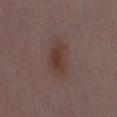{"biopsy_status": "not biopsied; imaged during a skin examination", "image": {"source": "total-body photography crop", "field_of_view_mm": 15}, "site": "front of the torso", "patient": {"sex": "female", "age_approx": 30}}